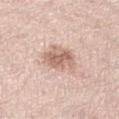* biopsy status: total-body-photography surveillance lesion; no biopsy
* lighting: white-light
* subject: female, aged around 65
* location: the right lower leg
* image source: total-body-photography crop, ~15 mm field of view
* lesion size: ~4 mm (longest diameter)